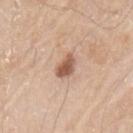Q: Was this lesion biopsied?
A: total-body-photography surveillance lesion; no biopsy
Q: Where on the body is the lesion?
A: the left upper arm
Q: What is the imaging modality?
A: ~15 mm crop, total-body skin-cancer survey
Q: How was the tile lit?
A: white-light
Q: What is the lesion's diameter?
A: about 3 mm
Q: What are the patient's age and sex?
A: male, about 65 years old
Q: What did automated image analysis measure?
A: a mean CIELAB color near L≈56 a*≈21 b*≈30; a nevus-likeness score of about 80/100 and a detector confidence of about 100 out of 100 that the crop contains a lesion